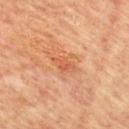{
  "biopsy_status": "not biopsied; imaged during a skin examination",
  "lighting": "cross-polarized",
  "image": {
    "source": "total-body photography crop",
    "field_of_view_mm": 15
  },
  "automated_metrics": {
    "border_irregularity_0_10": 3.5,
    "color_variation_0_10": 1.5,
    "peripheral_color_asymmetry": 0.5,
    "nevus_likeness_0_100": 5,
    "lesion_detection_confidence_0_100": 100
  },
  "patient": {
    "sex": "male",
    "age_approx": 65
  },
  "lesion_size": {
    "long_diameter_mm_approx": 3.0
  },
  "site": "mid back"
}